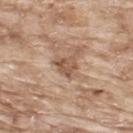Clinical summary:
A male subject in their mid- to late 60s. A close-up tile cropped from a whole-body skin photograph, about 15 mm across. The lesion is on the upper back.